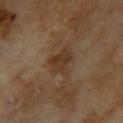workup: total-body-photography surveillance lesion; no biopsy | subject: female, approximately 60 years of age | illumination: cross-polarized | size: ~3.5 mm (longest diameter) | site: the upper back | acquisition: ~15 mm crop, total-body skin-cancer survey.